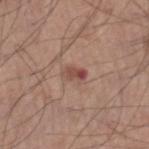This lesion was catalogued during total-body skin photography and was not selected for biopsy.
A 15 mm crop from a total-body photograph taken for skin-cancer surveillance.
The lesion is located on the leg.
The subject is a male aged 53 to 57.